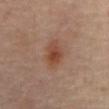Assessment: Captured during whole-body skin photography for melanoma surveillance; the lesion was not biopsied.